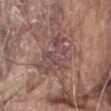Part of a total-body skin-imaging series; this lesion was reviewed on a skin check and was not flagged for biopsy.
Approximately 5.5 mm at its widest.
The tile uses white-light illumination.
From the front of the torso.
A 15 mm crop from a total-body photograph taken for skin-cancer surveillance.
A male subject, about 80 years old.
The lesion-visualizer software estimated a shape eccentricity near 0.8 and a symmetry-axis asymmetry near 0.35. And it measured a lesion color around L≈47 a*≈18 b*≈19 in CIELAB and a lesion-to-skin contrast of about 6.5 (normalized; higher = more distinct). It also reported a classifier nevus-likeness of about 0/100 and a detector confidence of about 65 out of 100 that the crop contains a lesion.